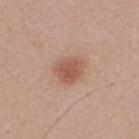Q: Was a biopsy performed?
A: no biopsy performed (imaged during a skin exam)
Q: Lesion location?
A: the upper back
Q: Patient demographics?
A: male, in their 40s
Q: What is the imaging modality?
A: total-body-photography crop, ~15 mm field of view